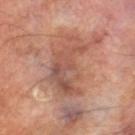This lesion was catalogued during total-body skin photography and was not selected for biopsy. Automated image analysis of the tile measured about 9 CIELAB-L* units darker than the surrounding skin and a lesion-to-skin contrast of about 6.5 (normalized; higher = more distinct). It also reported a border-irregularity index near 7/10, a color-variation rating of about 6/10, and a peripheral color-asymmetry measure near 2. And it measured an automated nevus-likeness rating near 0 out of 100 and a lesion-detection confidence of about 90/100. This image is a 15 mm lesion crop taken from a total-body photograph. From the left thigh. About 7 mm across. This is a cross-polarized tile. A male subject in their 70s.